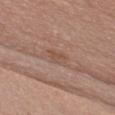Assessment: The lesion was photographed on a routine skin check and not biopsied; there is no pathology result. Image and clinical context: The lesion-visualizer software estimated a border-irregularity rating of about 4/10, a within-lesion color-variation index near 1.5/10, and peripheral color asymmetry of about 0.5. It also reported a nevus-likeness score of about 0/100 and a detector confidence of about 100 out of 100 that the crop contains a lesion. A female subject, roughly 50 years of age. A lesion tile, about 15 mm wide, cut from a 3D total-body photograph. Located on the chest. Captured under white-light illumination.